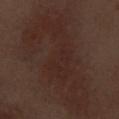workup: no biopsy performed (imaged during a skin exam)
acquisition: 15 mm crop, total-body photography
size: about 14.5 mm
tile lighting: white-light illumination
subject: male, aged 68 to 72
site: the left thigh